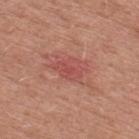| field | value |
|---|---|
| biopsy status | imaged on a skin check; not biopsied |